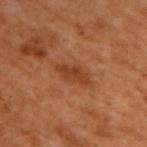{
  "biopsy_status": "not biopsied; imaged during a skin examination",
  "lighting": "cross-polarized",
  "lesion_size": {
    "long_diameter_mm_approx": 4.0
  },
  "automated_metrics": {
    "eccentricity": 0.9,
    "shape_asymmetry": 0.2,
    "cielab_L": 41,
    "cielab_a": 27,
    "cielab_b": 35,
    "vs_skin_darker_L": 8.0,
    "vs_skin_contrast_norm": 7.0,
    "color_variation_0_10": 2.5
  },
  "image": {
    "source": "total-body photography crop",
    "field_of_view_mm": 15
  },
  "patient": {
    "sex": "male",
    "age_approx": 50
  },
  "site": "upper back"
}